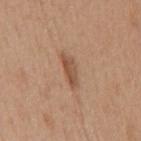Notes:
– follow-up — imaged on a skin check; not biopsied